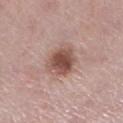biopsy_status: not biopsied; imaged during a skin examination
lesion_size:
  long_diameter_mm_approx: 4.0
lighting: white-light
automated_metrics:
  border_irregularity_0_10: 1.5
  nevus_likeness_0_100: 90
  lesion_detection_confidence_0_100: 100
patient:
  sex: female
  age_approx: 40
site: right lower leg
image:
  source: total-body photography crop
  field_of_view_mm: 15